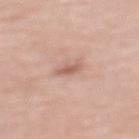{
  "biopsy_status": "not biopsied; imaged during a skin examination",
  "image": {
    "source": "total-body photography crop",
    "field_of_view_mm": 15
  },
  "automated_metrics": {
    "area_mm2_approx": 2.5,
    "eccentricity": 0.9,
    "shape_asymmetry": 0.3,
    "cielab_L": 59,
    "cielab_a": 22,
    "cielab_b": 28,
    "vs_skin_darker_L": 10.0,
    "nevus_likeness_0_100": 0
  },
  "lighting": "white-light",
  "patient": {
    "sex": "female",
    "age_approx": 70
  },
  "lesion_size": {
    "long_diameter_mm_approx": 2.5
  },
  "site": "mid back"
}